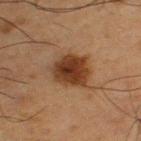Part of a total-body skin-imaging series; this lesion was reviewed on a skin check and was not flagged for biopsy. A roughly 15 mm field-of-view crop from a total-body skin photograph. On the left thigh. A male subject about 65 years old.